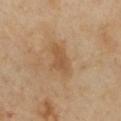workup: no biopsy performed (imaged during a skin exam)
subject: female, aged approximately 40
anatomic site: the chest
imaging modality: ~15 mm tile from a whole-body skin photo
size: about 4.5 mm
lighting: cross-polarized illumination